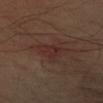The lesion was photographed on a routine skin check and not biopsied; there is no pathology result. A close-up tile cropped from a whole-body skin photograph, about 15 mm across. The tile uses cross-polarized illumination. The lesion is located on the left upper arm. The lesion's longest dimension is about 3.5 mm. A male patient, approximately 40 years of age. An algorithmic analysis of the crop reported an average lesion color of about L≈28 a*≈19 b*≈21 (CIELAB), about 5 CIELAB-L* units darker than the surrounding skin, and a normalized border contrast of about 5.5.